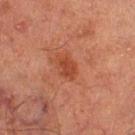Q: Was this lesion biopsied?
A: no biopsy performed (imaged during a skin exam)
Q: How large is the lesion?
A: ~3 mm (longest diameter)
Q: Automated lesion metrics?
A: a shape-asymmetry score of about 0.25 (0 = symmetric); a lesion color around L≈36 a*≈25 b*≈29 in CIELAB, a lesion–skin lightness drop of about 7, and a lesion-to-skin contrast of about 6.5 (normalized; higher = more distinct); internal color variation of about 1.5 on a 0–10 scale and a peripheral color-asymmetry measure near 0.5
Q: What is the imaging modality?
A: total-body-photography crop, ~15 mm field of view
Q: What lighting was used for the tile?
A: cross-polarized illumination
Q: Lesion location?
A: the left thigh
Q: What are the patient's age and sex?
A: male, about 65 years old